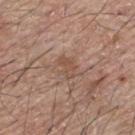| key | value |
|---|---|
| workup | total-body-photography surveillance lesion; no biopsy |
| image | ~15 mm tile from a whole-body skin photo |
| site | the upper back |
| lesion diameter | ~3 mm (longest diameter) |
| TBP lesion metrics | internal color variation of about 1.5 on a 0–10 scale and peripheral color asymmetry of about 0.5 |
| subject | male, in their mid-60s |